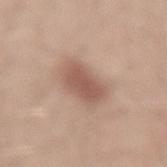On the lower back. A male subject, aged around 30. A region of skin cropped from a whole-body photographic capture, roughly 15 mm wide. Approximately 4.5 mm at its widest. Captured under white-light illumination.